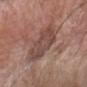Part of a total-body skin-imaging series; this lesion was reviewed on a skin check and was not flagged for biopsy.